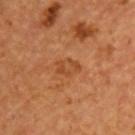notes=catalogued during a skin exam; not biopsied
imaging modality=~15 mm tile from a whole-body skin photo
anatomic site=the chest
patient=male, aged around 55